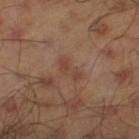Part of a total-body skin-imaging series; this lesion was reviewed on a skin check and was not flagged for biopsy. The subject is a male about 45 years old. The lesion is on the left thigh. A 15 mm crop from a total-body photograph taken for skin-cancer surveillance.